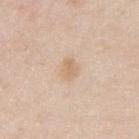The lesion was photographed on a routine skin check and not biopsied; there is no pathology result. Automated tile analysis of the lesion measured a shape eccentricity near 0.75 and a shape-asymmetry score of about 0.2 (0 = symmetric). The analysis additionally found a nevus-likeness score of about 5/100 and lesion-presence confidence of about 100/100. The patient is a male aged around 35. The lesion's longest dimension is about 2.5 mm. The lesion is located on the upper back. Imaged with white-light lighting. Cropped from a whole-body photographic skin survey; the tile spans about 15 mm.